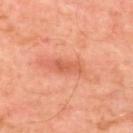Q: Where on the body is the lesion?
A: the back
Q: How was the tile lit?
A: cross-polarized
Q: Patient demographics?
A: male, about 50 years old
Q: What did automated image analysis measure?
A: an area of roughly 4.5 mm²; a border-irregularity index near 3/10 and a peripheral color-asymmetry measure near 0.5; a classifier nevus-likeness of about 0/100
Q: How was this image acquired?
A: 15 mm crop, total-body photography